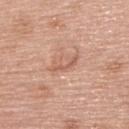This lesion was catalogued during total-body skin photography and was not selected for biopsy. The recorded lesion diameter is about 3.5 mm. Automated tile analysis of the lesion measured a lesion area of about 4 mm² and an outline eccentricity of about 0.95 (0 = round, 1 = elongated). The analysis additionally found a within-lesion color-variation index near 0/10 and a peripheral color-asymmetry measure near 0. And it measured a lesion-detection confidence of about 100/100. A female subject, approximately 60 years of age. On the upper back. This is a white-light tile. A lesion tile, about 15 mm wide, cut from a 3D total-body photograph.